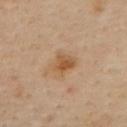workup: total-body-photography surveillance lesion; no biopsy | TBP lesion metrics: a lesion color around L≈55 a*≈19 b*≈37 in CIELAB, roughly 9 lightness units darker than nearby skin, and a lesion-to-skin contrast of about 7.5 (normalized; higher = more distinct) | site: the upper back | imaging modality: ~15 mm tile from a whole-body skin photo | tile lighting: cross-polarized | lesion diameter: about 2.5 mm | subject: female, aged approximately 60.